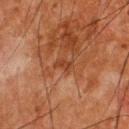Notes:
• biopsy status: catalogued during a skin exam; not biopsied
• lesion diameter: about 2.5 mm
• illumination: cross-polarized illumination
• location: the back
• imaging modality: total-body-photography crop, ~15 mm field of view
• subject: male, roughly 65 years of age